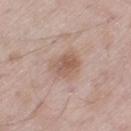notes: catalogued during a skin exam; not biopsied | acquisition: total-body-photography crop, ~15 mm field of view | subject: male, in their 60s | TBP lesion metrics: a detector confidence of about 100 out of 100 that the crop contains a lesion | location: the leg.